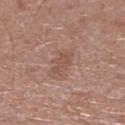notes = imaged on a skin check; not biopsied
image source = 15 mm crop, total-body photography
lighting = white-light
site = the left lower leg
patient = male, about 60 years old